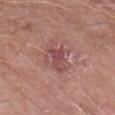Q: Is there a histopathology result?
A: total-body-photography surveillance lesion; no biopsy
Q: Lesion size?
A: ~4 mm (longest diameter)
Q: What did automated image analysis measure?
A: a footprint of about 9.5 mm² and two-axis asymmetry of about 0.35; a mean CIELAB color near L≈50 a*≈24 b*≈21, roughly 8 lightness units darker than nearby skin, and a lesion-to-skin contrast of about 6 (normalized; higher = more distinct); a lesion-detection confidence of about 100/100
Q: Illumination type?
A: white-light
Q: Lesion location?
A: the left forearm
Q: How was this image acquired?
A: 15 mm crop, total-body photography
Q: What are the patient's age and sex?
A: male, aged around 65